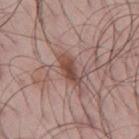No biopsy was performed on this lesion — it was imaged during a full skin examination and was not determined to be concerning.
A roughly 15 mm field-of-view crop from a total-body skin photograph.
Located on the mid back.
The subject is a male about 45 years old.